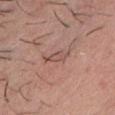Assessment:
The lesion was photographed on a routine skin check and not biopsied; there is no pathology result.
Clinical summary:
This image is a 15 mm lesion crop taken from a total-body photograph. A male patient, roughly 30 years of age. The tile uses white-light illumination. Located on the chest.